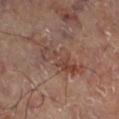Part of a total-body skin-imaging series; this lesion was reviewed on a skin check and was not flagged for biopsy.
Measured at roughly 6 mm in maximum diameter.
From the left lower leg.
The lesion-visualizer software estimated a lesion area of about 12 mm² and a shape-asymmetry score of about 0.4 (0 = symmetric).
This is a cross-polarized tile.
Cropped from a total-body skin-imaging series; the visible field is about 15 mm.